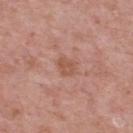illumination: white-light | anatomic site: the upper back | subject: male, in their mid- to late 60s | acquisition: ~15 mm tile from a whole-body skin photo | size: ~2.5 mm (longest diameter) | TBP lesion metrics: a lesion area of about 4.5 mm², an eccentricity of roughly 0.55, and two-axis asymmetry of about 0.3; a color-variation rating of about 1.5/10 and a peripheral color-asymmetry measure near 0.5.